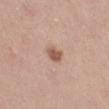| feature | finding |
|---|---|
| biopsy status | total-body-photography surveillance lesion; no biopsy |
| tile lighting | white-light |
| patient | female, approximately 20 years of age |
| site | the right thigh |
| image source | total-body-photography crop, ~15 mm field of view |
| automated metrics | a lesion color around L≈61 a*≈18 b*≈27 in CIELAB and a normalized border contrast of about 5.5; a border-irregularity index near 2/10 |